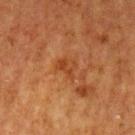Recorded during total-body skin imaging; not selected for excision or biopsy.
Located on the arm.
The subject is a female aged around 50.
Approximately 3 mm at its widest.
Automated image analysis of the tile measured an area of roughly 4.5 mm², an eccentricity of roughly 0.65, and a shape-asymmetry score of about 0.45 (0 = symmetric). And it measured border irregularity of about 4 on a 0–10 scale and radial color variation of about 1.
This is a cross-polarized tile.
A close-up tile cropped from a whole-body skin photograph, about 15 mm across.